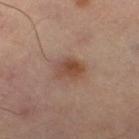The lesion-visualizer software estimated a border-irregularity index near 2/10, a color-variation rating of about 5.5/10, and a peripheral color-asymmetry measure near 2. It also reported a classifier nevus-likeness of about 75/100 and a lesion-detection confidence of about 100/100.
A 15 mm close-up tile from a total-body photography series done for melanoma screening.
On the leg.
The tile uses cross-polarized illumination.